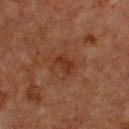biopsy status = total-body-photography surveillance lesion; no biopsy
subject = male, approximately 75 years of age
image source = total-body-photography crop, ~15 mm field of view
body site = the chest